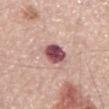Clinical impression:
No biopsy was performed on this lesion — it was imaged during a full skin examination and was not determined to be concerning.
Acquisition and patient details:
A 15 mm close-up tile from a total-body photography series done for melanoma screening. Imaged with white-light lighting. A male subject aged 68–72. Measured at roughly 3 mm in maximum diameter. The lesion is on the mid back.